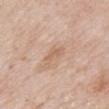| key | value |
|---|---|
| notes | imaged on a skin check; not biopsied |
| image | total-body-photography crop, ~15 mm field of view |
| anatomic site | the mid back |
| diameter | ≈2.5 mm |
| subject | male, in their mid-80s or older |
| automated metrics | an area of roughly 2.5 mm², an outline eccentricity of about 0.9 (0 = round, 1 = elongated), and a symmetry-axis asymmetry near 0.4; a lesion color around L≈62 a*≈18 b*≈32 in CIELAB and a normalized border contrast of about 5; a border-irregularity rating of about 4/10, a within-lesion color-variation index near 0/10, and a peripheral color-asymmetry measure near 0; a nevus-likeness score of about 0/100 and a detector confidence of about 100 out of 100 that the crop contains a lesion |